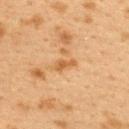Assessment: Captured during whole-body skin photography for melanoma surveillance; the lesion was not biopsied. Acquisition and patient details: The tile uses cross-polarized illumination. A female subject, approximately 40 years of age. A 15 mm crop from a total-body photograph taken for skin-cancer surveillance. From the upper back. An algorithmic analysis of the crop reported a lesion area of about 3 mm², an outline eccentricity of about 0.9 (0 = round, 1 = elongated), and two-axis asymmetry of about 0.45. It also reported roughly 8 lightness units darker than nearby skin and a normalized lesion–skin contrast near 7. And it measured a classifier nevus-likeness of about 0/100 and a lesion-detection confidence of about 100/100. The recorded lesion diameter is about 2.5 mm.